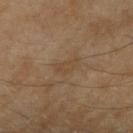Q: Was this lesion biopsied?
A: catalogued during a skin exam; not biopsied
Q: Patient demographics?
A: female, aged 58–62
Q: Illumination type?
A: cross-polarized
Q: How was this image acquired?
A: ~15 mm tile from a whole-body skin photo
Q: Lesion location?
A: the arm
Q: What is the lesion's diameter?
A: ≈3 mm